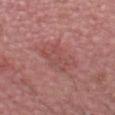Assessment: Recorded during total-body skin imaging; not selected for excision or biopsy. Clinical summary: A roughly 15 mm field-of-view crop from a total-body skin photograph. The patient is a male roughly 30 years of age.